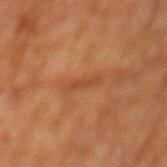{"biopsy_status": "not biopsied; imaged during a skin examination", "site": "back", "patient": {"sex": "male", "age_approx": 80}, "image": {"source": "total-body photography crop", "field_of_view_mm": 15}, "automated_metrics": {"cielab_L": 36, "cielab_a": 21, "cielab_b": 30, "vs_skin_darker_L": 5.0, "vs_skin_contrast_norm": 5.0, "border_irregularity_0_10": 3.0, "peripheral_color_asymmetry": 0.0}, "lighting": "cross-polarized"}